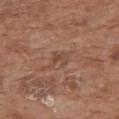Context:
From the upper back. A close-up tile cropped from a whole-body skin photograph, about 15 mm across. The patient is a female about 75 years old.